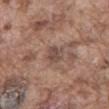Part of a total-body skin-imaging series; this lesion was reviewed on a skin check and was not flagged for biopsy.
From the abdomen.
The subject is a male roughly 75 years of age.
Approximately 2.5 mm at its widest.
A roughly 15 mm field-of-view crop from a total-body skin photograph.
An algorithmic analysis of the crop reported an area of roughly 4 mm² and a shape eccentricity near 0.7. It also reported an average lesion color of about L≈47 a*≈17 b*≈23 (CIELAB) and a normalized border contrast of about 7. The analysis additionally found internal color variation of about 1.5 on a 0–10 scale and a peripheral color-asymmetry measure near 0.5. The analysis additionally found an automated nevus-likeness rating near 0 out of 100 and lesion-presence confidence of about 100/100.
The tile uses white-light illumination.